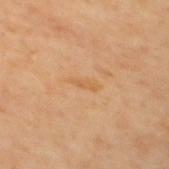notes=catalogued during a skin exam; not biopsied | patient=female, aged 63 to 67 | image=~15 mm crop, total-body skin-cancer survey | anatomic site=the mid back.